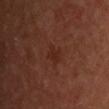About 2.5 mm across.
Cropped from a total-body skin-imaging series; the visible field is about 15 mm.
The subject is a male in their 50s.
On the chest.